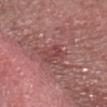Q: Is there a histopathology result?
A: total-body-photography surveillance lesion; no biopsy
Q: Automated lesion metrics?
A: a lesion area of about 4 mm² and two-axis asymmetry of about 0.3; a mean CIELAB color near L≈44 a*≈27 b*≈21
Q: What is the lesion's diameter?
A: ~2.5 mm (longest diameter)
Q: What is the anatomic site?
A: the head or neck
Q: How was this image acquired?
A: ~15 mm tile from a whole-body skin photo
Q: Who is the patient?
A: male, aged around 60